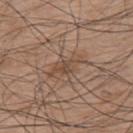Captured under white-light illumination.
From the back.
Approximately 4.5 mm at its widest.
A close-up tile cropped from a whole-body skin photograph, about 15 mm across.
A male patient aged 73 to 77.